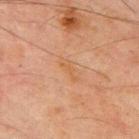| field | value |
|---|---|
| biopsy status | no biopsy performed (imaged during a skin exam) |
| imaging modality | 15 mm crop, total-body photography |
| site | the chest |
| tile lighting | cross-polarized |
| patient | male, roughly 70 years of age |